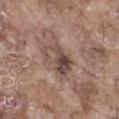Q: Was this lesion biopsied?
A: no biopsy performed (imaged during a skin exam)
Q: What are the patient's age and sex?
A: male, in their mid-70s
Q: What kind of image is this?
A: 15 mm crop, total-body photography
Q: How large is the lesion?
A: about 4.5 mm
Q: What did automated image analysis measure?
A: an outline eccentricity of about 0.8 (0 = round, 1 = elongated) and a symmetry-axis asymmetry near 0.5; a mean CIELAB color near L≈46 a*≈16 b*≈22 and a lesion–skin lightness drop of about 11; internal color variation of about 5 on a 0–10 scale and peripheral color asymmetry of about 2
Q: Illumination type?
A: white-light
Q: Where on the body is the lesion?
A: the front of the torso